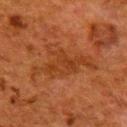- notes: imaged on a skin check; not biopsied